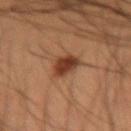Assessment:
Captured during whole-body skin photography for melanoma surveillance; the lesion was not biopsied.
Acquisition and patient details:
A male subject aged around 35. A 15 mm close-up tile from a total-body photography series done for melanoma screening. On the right forearm.